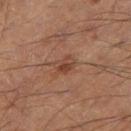The lesion was tiled from a total-body skin photograph and was not biopsied. A male subject, aged 53–57. From the right thigh. Cropped from a total-body skin-imaging series; the visible field is about 15 mm.